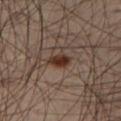{"biopsy_status": "not biopsied; imaged during a skin examination", "patient": {"sex": "male", "age_approx": 45}, "site": "right thigh", "image": {"source": "total-body photography crop", "field_of_view_mm": 15}}